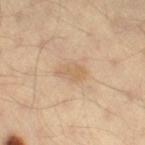Q: Was this lesion biopsied?
A: imaged on a skin check; not biopsied
Q: Lesion location?
A: the right thigh
Q: What is the imaging modality?
A: total-body-photography crop, ~15 mm field of view
Q: Lesion size?
A: ~3.5 mm (longest diameter)
Q: Who is the patient?
A: male, in their 40s
Q: Automated lesion metrics?
A: a footprint of about 5 mm², a shape eccentricity near 0.8, and a shape-asymmetry score of about 0.25 (0 = symmetric); a mean CIELAB color near L≈63 a*≈16 b*≈34, roughly 7 lightness units darker than nearby skin, and a normalized border contrast of about 5; a lesion-detection confidence of about 100/100
Q: Illumination type?
A: cross-polarized illumination